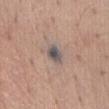Recorded during total-body skin imaging; not selected for excision or biopsy. The recorded lesion diameter is about 2.5 mm. Captured under white-light illumination. The lesion is located on the abdomen. Automated image analysis of the tile measured a lesion color around L≈52 a*≈9 b*≈16 in CIELAB, a lesion–skin lightness drop of about 12, and a normalized lesion–skin contrast near 10. The software also gave border irregularity of about 2 on a 0–10 scale and radial color variation of about 1.5. The software also gave a classifier nevus-likeness of about 40/100 and a lesion-detection confidence of about 95/100. A 15 mm close-up tile from a total-body photography series done for melanoma screening. A male subject aged around 70.